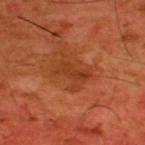Q: Was a biopsy performed?
A: imaged on a skin check; not biopsied
Q: Where on the body is the lesion?
A: the upper back
Q: What is the lesion's diameter?
A: about 4 mm
Q: What are the patient's age and sex?
A: male, aged approximately 60
Q: What is the imaging modality?
A: total-body-photography crop, ~15 mm field of view
Q: Illumination type?
A: cross-polarized illumination